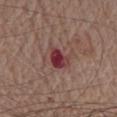No biopsy was performed on this lesion — it was imaged during a full skin examination and was not determined to be concerning.
On the chest.
The lesion's longest dimension is about 3 mm.
Captured under white-light illumination.
An algorithmic analysis of the crop reported a border-irregularity index near 3.5/10, a color-variation rating of about 6.5/10, and peripheral color asymmetry of about 2. And it measured a nevus-likeness score of about 0/100.
A region of skin cropped from a whole-body photographic capture, roughly 15 mm wide.
A male subject, in their mid-70s.